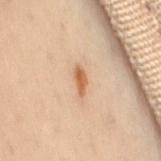Q: Is there a histopathology result?
A: no biopsy performed (imaged during a skin exam)
Q: Where on the body is the lesion?
A: the lower back
Q: Patient demographics?
A: female, roughly 50 years of age
Q: What kind of image is this?
A: ~15 mm tile from a whole-body skin photo